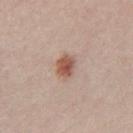Impression:
The lesion was tiled from a total-body skin photograph and was not biopsied.
Acquisition and patient details:
On the front of the torso. The subject is a male aged 58–62. This image is a 15 mm lesion crop taken from a total-body photograph.